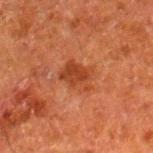Notes:
- workup · total-body-photography surveillance lesion; no biopsy
- patient · male, about 80 years old
- body site · the leg
- image source · total-body-photography crop, ~15 mm field of view